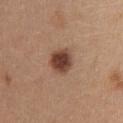Part of a total-body skin-imaging series; this lesion was reviewed on a skin check and was not flagged for biopsy.
About 3.5 mm across.
Cropped from a whole-body photographic skin survey; the tile spans about 15 mm.
Captured under white-light illumination.
From the upper back.
A female subject, aged approximately 25.